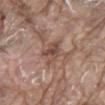Q: How was this image acquired?
A: total-body-photography crop, ~15 mm field of view
Q: Who is the patient?
A: male, aged around 80
Q: What is the anatomic site?
A: the mid back